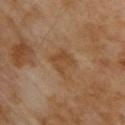The lesion was tiled from a total-body skin photograph and was not biopsied. This is a cross-polarized tile. Located on the back. A region of skin cropped from a whole-body photographic capture, roughly 15 mm wide. A male subject about 70 years old. The recorded lesion diameter is about 3.5 mm.